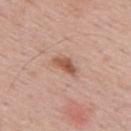Imaged during a routine full-body skin examination; the lesion was not biopsied and no histopathology is available.
On the upper back.
A 15 mm close-up tile from a total-body photography series done for melanoma screening.
A male subject about 55 years old.
Captured under white-light illumination.
Longest diameter approximately 3 mm.